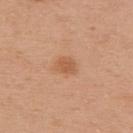  site: upper back
  patient:
    sex: female
    age_approx: 40
  lesion_size:
    long_diameter_mm_approx: 2.5
  image:
    source: total-body photography crop
    field_of_view_mm: 15